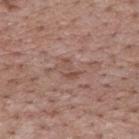follow-up — catalogued during a skin exam; not biopsied
imaging modality — total-body-photography crop, ~15 mm field of view
patient — male, aged approximately 70
location — the back
image-analysis metrics — a classifier nevus-likeness of about 0/100